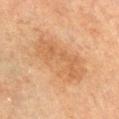{
  "biopsy_status": "not biopsied; imaged during a skin examination",
  "patient": {
    "sex": "male",
    "age_approx": 75
  },
  "image": {
    "source": "total-body photography crop",
    "field_of_view_mm": 15
  },
  "site": "left upper arm"
}